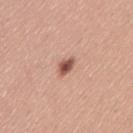Assessment:
The lesion was photographed on a routine skin check and not biopsied; there is no pathology result.
Image and clinical context:
A 15 mm crop from a total-body photograph taken for skin-cancer surveillance. Measured at roughly 2.5 mm in maximum diameter. A female patient aged 28–32. Captured under white-light illumination. The total-body-photography lesion software estimated a lesion area of about 3 mm², an eccentricity of roughly 0.8, and a shape-asymmetry score of about 0.2 (0 = symmetric). And it measured a lesion–skin lightness drop of about 16. It also reported a classifier nevus-likeness of about 95/100 and a detector confidence of about 100 out of 100 that the crop contains a lesion. The lesion is on the back.